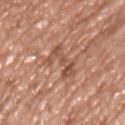workup = no biopsy performed (imaged during a skin exam) | location = the upper back | subject = male, approximately 50 years of age | tile lighting = white-light illumination | lesion diameter = ≈4 mm | image = total-body-photography crop, ~15 mm field of view.